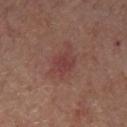{"biopsy_status": "not biopsied; imaged during a skin examination", "image": {"source": "total-body photography crop", "field_of_view_mm": 15}, "patient": {"sex": "male", "age_approx": 55}, "lighting": "white-light", "site": "chest", "automated_metrics": {"cielab_L": 40, "cielab_a": 25, "cielab_b": 22, "vs_skin_darker_L": 7.0}, "lesion_size": {"long_diameter_mm_approx": 3.0}}